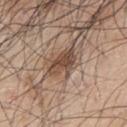follow-up=no biopsy performed (imaged during a skin exam) | image source=~15 mm tile from a whole-body skin photo | patient=male, aged approximately 70 | automated lesion analysis=a symmetry-axis asymmetry near 0.3; a lesion color around L≈47 a*≈17 b*≈26 in CIELAB, a lesion–skin lightness drop of about 12, and a normalized lesion–skin contrast near 9; a nevus-likeness score of about 75/100 and a detector confidence of about 100 out of 100 that the crop contains a lesion | lighting=white-light | lesion size=≈4 mm | site=the left upper arm.